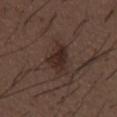Case summary:
- workup · catalogued during a skin exam; not biopsied
- subject · male, in their 50s
- imaging modality · ~15 mm crop, total-body skin-cancer survey
- body site · the abdomen